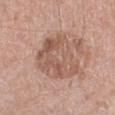biopsy status — catalogued during a skin exam; not biopsied
tile lighting — white-light
patient — male, in their 50s
anatomic site — the leg
lesion size — ~6 mm (longest diameter)
TBP lesion metrics — a lesion area of about 22 mm², an eccentricity of roughly 0.65, and a symmetry-axis asymmetry near 0.35; a border-irregularity rating of about 4/10, a color-variation rating of about 6/10, and peripheral color asymmetry of about 2; a classifier nevus-likeness of about 0/100
image source — 15 mm crop, total-body photography